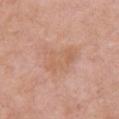A female patient aged approximately 60.
An algorithmic analysis of the crop reported an area of roughly 11 mm², an outline eccentricity of about 0.8 (0 = round, 1 = elongated), and two-axis asymmetry of about 0.4. The analysis additionally found a mean CIELAB color near L≈61 a*≈21 b*≈32, a lesion–skin lightness drop of about 6, and a normalized lesion–skin contrast near 5.
The tile uses white-light illumination.
A roughly 15 mm field-of-view crop from a total-body skin photograph.
Located on the chest.
Approximately 4.5 mm at its widest.